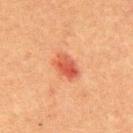The lesion was photographed on a routine skin check and not biopsied; there is no pathology result.
On the mid back.
A male subject about 40 years old.
A 15 mm close-up extracted from a 3D total-body photography capture.